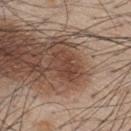Recorded during total-body skin imaging; not selected for excision or biopsy.
An algorithmic analysis of the crop reported a lesion–skin lightness drop of about 8 and a normalized border contrast of about 6.5. The software also gave a classifier nevus-likeness of about 10/100.
Captured under white-light illumination.
A 15 mm crop from a total-body photograph taken for skin-cancer surveillance.
The subject is a male aged around 45.
Approximately 4 mm at its widest.
From the back.